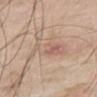Recorded during total-body skin imaging; not selected for excision or biopsy.
Automated image analysis of the tile measured an average lesion color of about L≈61 a*≈17 b*≈26 (CIELAB), roughly 7 lightness units darker than nearby skin, and a normalized border contrast of about 4.5. The software also gave a within-lesion color-variation index near 8/10 and radial color variation of about 2.5. It also reported a classifier nevus-likeness of about 0/100 and lesion-presence confidence of about 95/100.
The tile uses white-light illumination.
Located on the right thigh.
The recorded lesion diameter is about 6.5 mm.
A roughly 15 mm field-of-view crop from a total-body skin photograph.
A male subject, aged around 70.